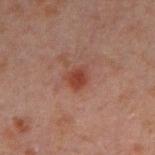Assessment:
Imaged during a routine full-body skin examination; the lesion was not biopsied and no histopathology is available.
Acquisition and patient details:
Cropped from a whole-body photographic skin survey; the tile spans about 15 mm. The lesion is located on the right forearm. A male subject, in their 30s. The lesion's longest dimension is about 2.5 mm. This is a cross-polarized tile.